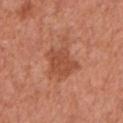Case summary:
* notes: imaged on a skin check; not biopsied
* lesion diameter: ≈5 mm
* anatomic site: the left upper arm
* image: ~15 mm tile from a whole-body skin photo
* subject: male, about 65 years old